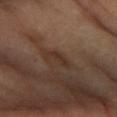Q: Was this lesion biopsied?
A: no biopsy performed (imaged during a skin exam)
Q: What did automated image analysis measure?
A: a lesion area of about 4 mm² and a shape-asymmetry score of about 0.5 (0 = symmetric); border irregularity of about 6 on a 0–10 scale and internal color variation of about 0 on a 0–10 scale
Q: Lesion size?
A: ≈3.5 mm
Q: Illumination type?
A: cross-polarized illumination
Q: What is the anatomic site?
A: the arm
Q: How was this image acquired?
A: ~15 mm crop, total-body skin-cancer survey
Q: What are the patient's age and sex?
A: female, aged approximately 70